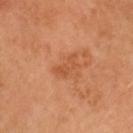This lesion was catalogued during total-body skin photography and was not selected for biopsy.
Imaged with cross-polarized lighting.
Longest diameter approximately 3.5 mm.
From the head or neck.
A male patient, aged approximately 45.
A 15 mm crop from a total-body photograph taken for skin-cancer surveillance.